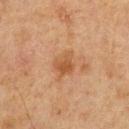notes — total-body-photography surveillance lesion; no biopsy
illumination — cross-polarized
location — the chest
patient — male, about 75 years old
image source — ~15 mm crop, total-body skin-cancer survey
TBP lesion metrics — a mean CIELAB color near L≈43 a*≈19 b*≈32, roughly 7 lightness units darker than nearby skin, and a normalized lesion–skin contrast near 7; a nevus-likeness score of about 10/100 and a detector confidence of about 100 out of 100 that the crop contains a lesion
lesion diameter — ≈3 mm